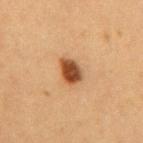| field | value |
|---|---|
| workup | catalogued during a skin exam; not biopsied |
| imaging modality | total-body-photography crop, ~15 mm field of view |
| diameter | ~3 mm (longest diameter) |
| lighting | cross-polarized |
| body site | the mid back |
| patient | female, aged 38 to 42 |